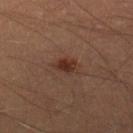Part of a total-body skin-imaging series; this lesion was reviewed on a skin check and was not flagged for biopsy.
An algorithmic analysis of the crop reported a border-irregularity rating of about 2.5/10, a color-variation rating of about 2.5/10, and a peripheral color-asymmetry measure near 0.5.
A 15 mm crop from a total-body photograph taken for skin-cancer surveillance.
Measured at roughly 3 mm in maximum diameter.
The patient is a male in their 40s.
On the right lower leg.
Imaged with cross-polarized lighting.